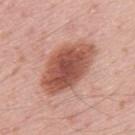This lesion was catalogued during total-body skin photography and was not selected for biopsy.